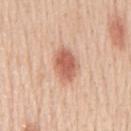notes: catalogued during a skin exam; not biopsied
lesion size: about 4.5 mm
subject: male, aged 58 to 62
acquisition: ~15 mm tile from a whole-body skin photo
TBP lesion metrics: a lesion area of about 9.5 mm², an eccentricity of roughly 0.8, and two-axis asymmetry of about 0.15
site: the mid back
illumination: white-light